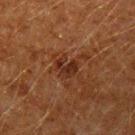The lesion was tiled from a total-body skin photograph and was not biopsied. The patient is a male aged approximately 60. A region of skin cropped from a whole-body photographic capture, roughly 15 mm wide. The lesion is on the left upper arm.